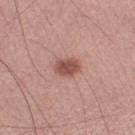Clinical impression:
Recorded during total-body skin imaging; not selected for excision or biopsy.
Acquisition and patient details:
Imaged with white-light lighting. Automated image analysis of the tile measured a footprint of about 6 mm², a shape eccentricity near 0.7, and two-axis asymmetry of about 0.2. It also reported an automated nevus-likeness rating near 95 out of 100. From the right lower leg. A male patient, aged around 50. Longest diameter approximately 3 mm. A 15 mm close-up extracted from a 3D total-body photography capture.